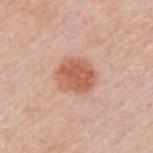Imaged during a routine full-body skin examination; the lesion was not biopsied and no histopathology is available. A region of skin cropped from a whole-body photographic capture, roughly 15 mm wide. From the upper back. An algorithmic analysis of the crop reported a lesion area of about 12 mm², an outline eccentricity of about 0.5 (0 = round, 1 = elongated), and a shape-asymmetry score of about 0.1 (0 = symmetric). The software also gave a border-irregularity rating of about 1/10 and a peripheral color-asymmetry measure near 1. The subject is a male aged 58–62.